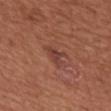Assessment: This lesion was catalogued during total-body skin photography and was not selected for biopsy. Context: On the upper back. The patient is a female aged around 75. A close-up tile cropped from a whole-body skin photograph, about 15 mm across. The recorded lesion diameter is about 3 mm.